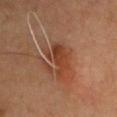Q: Was this lesion biopsied?
A: total-body-photography surveillance lesion; no biopsy
Q: Where on the body is the lesion?
A: the front of the torso
Q: How large is the lesion?
A: about 4.5 mm
Q: What is the imaging modality?
A: ~15 mm tile from a whole-body skin photo
Q: Patient demographics?
A: male, roughly 45 years of age
Q: What did automated image analysis measure?
A: roughly 8 lightness units darker than nearby skin; a border-irregularity rating of about 6/10, internal color variation of about 4.5 on a 0–10 scale, and peripheral color asymmetry of about 1.5